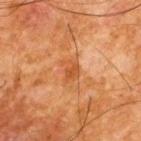  biopsy_status: not biopsied; imaged during a skin examination
  image:
    source: total-body photography crop
    field_of_view_mm: 15
  site: upper back
  patient:
    sex: male
    age_approx: 65